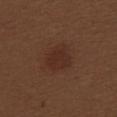Assessment: The lesion was photographed on a routine skin check and not biopsied; there is no pathology result. Acquisition and patient details: The tile uses white-light illumination. The patient is a female approximately 30 years of age. Located on the upper back. A lesion tile, about 15 mm wide, cut from a 3D total-body photograph.